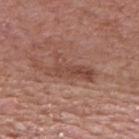- biopsy status: no biopsy performed (imaged during a skin exam)
- subject: male, in their mid-60s
- acquisition: total-body-photography crop, ~15 mm field of view
- site: the mid back
- size: about 5.5 mm
- lighting: white-light